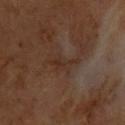Assessment: The lesion was tiled from a total-body skin photograph and was not biopsied. Clinical summary: A 15 mm crop from a total-body photograph taken for skin-cancer surveillance. This is a cross-polarized tile. A male patient, aged around 60. About 4 mm across. On the chest.